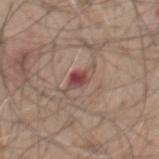Q: Is there a histopathology result?
A: catalogued during a skin exam; not biopsied
Q: Where on the body is the lesion?
A: the chest
Q: How was this image acquired?
A: ~15 mm crop, total-body skin-cancer survey
Q: How large is the lesion?
A: ~4 mm (longest diameter)
Q: How was the tile lit?
A: white-light
Q: Who is the patient?
A: male, approximately 75 years of age